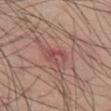The lesion was tiled from a total-body skin photograph and was not biopsied.
Longest diameter approximately 3 mm.
This image is a 15 mm lesion crop taken from a total-body photograph.
The total-body-photography lesion software estimated an eccentricity of roughly 0.7 and two-axis asymmetry of about 0.5. And it measured an average lesion color of about L≈49 a*≈26 b*≈23 (CIELAB), about 7 CIELAB-L* units darker than the surrounding skin, and a normalized lesion–skin contrast near 5.5. The analysis additionally found a border-irregularity rating of about 6/10 and internal color variation of about 1.5 on a 0–10 scale.
On the left thigh.
Captured under white-light illumination.
The subject is a male approximately 55 years of age.